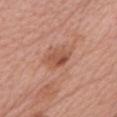Assessment: The lesion was tiled from a total-body skin photograph and was not biopsied. Background: The patient is a female aged 58 to 62. This is a white-light tile. A 15 mm close-up extracted from a 3D total-body photography capture. From the front of the torso. About 3.5 mm across.